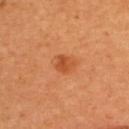Recorded during total-body skin imaging; not selected for excision or biopsy. Longest diameter approximately 2.5 mm. Captured under cross-polarized illumination. On the upper back. This image is a 15 mm lesion crop taken from a total-body photograph. The total-body-photography lesion software estimated a lesion area of about 4 mm² and a shape-asymmetry score of about 0.25 (0 = symmetric). And it measured an average lesion color of about L≈51 a*≈31 b*≈42 (CIELAB) and a lesion–skin lightness drop of about 9. A female subject roughly 35 years of age.